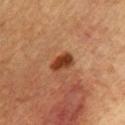notes — no biopsy performed (imaged during a skin exam) | anatomic site — the chest | image-analysis metrics — a lesion area of about 5 mm² and an eccentricity of roughly 0.75; a classifier nevus-likeness of about 95/100 and lesion-presence confidence of about 100/100 | illumination — cross-polarized | lesion diameter — ≈3 mm | imaging modality — ~15 mm tile from a whole-body skin photo | subject — male, aged around 60.